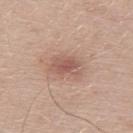  biopsy_status: not biopsied; imaged during a skin examination
  lesion_size:
    long_diameter_mm_approx: 4.0
  automated_metrics:
    cielab_L: 56
    cielab_a: 20
    cielab_b: 26
    vs_skin_darker_L: 10.0
    vs_skin_contrast_norm: 6.5
    peripheral_color_asymmetry: 1.0
    nevus_likeness_0_100: 45
  patient:
    sex: male
    age_approx: 25
  lighting: white-light
  site: upper back
  image:
    source: total-body photography crop
    field_of_view_mm: 15